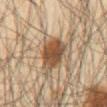| feature | finding |
|---|---|
| follow-up | no biopsy performed (imaged during a skin exam) |
| site | the chest |
| acquisition | total-body-photography crop, ~15 mm field of view |
| subject | male, in their mid- to late 60s |
| diameter | ~4.5 mm (longest diameter) |
| TBP lesion metrics | an average lesion color of about L≈40 a*≈14 b*≈27 (CIELAB), about 12 CIELAB-L* units darker than the surrounding skin, and a lesion-to-skin contrast of about 10 (normalized; higher = more distinct); a color-variation rating of about 4.5/10 |